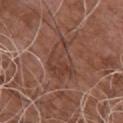Case summary:
* biopsy status · imaged on a skin check; not biopsied
* location · the chest
* automated lesion analysis · an area of roughly 13 mm², a shape eccentricity near 0.8, and two-axis asymmetry of about 0.35; roughly 6 lightness units darker than nearby skin and a lesion-to-skin contrast of about 5.5 (normalized; higher = more distinct); border irregularity of about 4.5 on a 0–10 scale, internal color variation of about 4.5 on a 0–10 scale, and a peripheral color-asymmetry measure near 2; a classifier nevus-likeness of about 0/100 and lesion-presence confidence of about 85/100
* illumination · white-light illumination
* subject · male, aged 73 to 77
* lesion size · about 5.5 mm
* image · ~15 mm tile from a whole-body skin photo